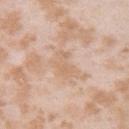notes = no biopsy performed (imaged during a skin exam); imaging modality = 15 mm crop, total-body photography; patient = female, about 25 years old; site = the arm; lesion diameter = ≈4 mm.